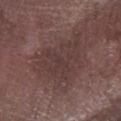Context:
A male subject aged approximately 75. A 15 mm close-up extracted from a 3D total-body photography capture. Imaged with white-light lighting. About 7.5 mm across. On the right lower leg.